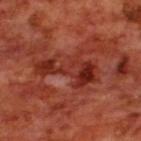Case summary:
– notes · total-body-photography surveillance lesion; no biopsy
– subject · male, roughly 70 years of age
– automated metrics · a lesion–skin lightness drop of about 9 and a normalized lesion–skin contrast near 8; a border-irregularity rating of about 9/10 and internal color variation of about 5.5 on a 0–10 scale; a nevus-likeness score of about 0/100
– acquisition · total-body-photography crop, ~15 mm field of view
– size · ~7 mm (longest diameter)
– tile lighting · cross-polarized illumination
– site · the upper back